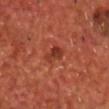The lesion was tiled from a total-body skin photograph and was not biopsied. A 15 mm close-up tile from a total-body photography series done for melanoma screening. Captured under cross-polarized illumination. Automated image analysis of the tile measured roughly 9 lightness units darker than nearby skin. The analysis additionally found an automated nevus-likeness rating near 5 out of 100. Located on the chest. The subject is a male aged around 70.